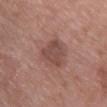{
  "biopsy_status": "not biopsied; imaged during a skin examination",
  "lighting": "white-light",
  "site": "upper back",
  "lesion_size": {
    "long_diameter_mm_approx": 4.0
  },
  "image": {
    "source": "total-body photography crop",
    "field_of_view_mm": 15
  },
  "patient": {
    "sex": "female",
    "age_approx": 65
  }
}